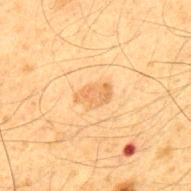Findings:
* biopsy status: no biopsy performed (imaged during a skin exam)
* image source: ~15 mm tile from a whole-body skin photo
* subject: male, about 65 years old
* lighting: cross-polarized illumination
* anatomic site: the upper back